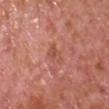Impression: Recorded during total-body skin imaging; not selected for excision or biopsy. Image and clinical context: Automated tile analysis of the lesion measured a mean CIELAB color near L≈52 a*≈28 b*≈30, roughly 7 lightness units darker than nearby skin, and a normalized border contrast of about 5.5. And it measured an automated nevus-likeness rating near 0 out of 100. Located on the chest. The tile uses white-light illumination. A male patient aged 63 to 67. A 15 mm crop from a total-body photograph taken for skin-cancer surveillance.